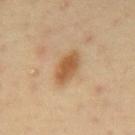follow-up = no biopsy performed (imaged during a skin exam)
lesion diameter = about 4.5 mm
site = the mid back
image-analysis metrics = a within-lesion color-variation index near 3/10; an automated nevus-likeness rating near 90 out of 100 and a lesion-detection confidence of about 100/100
image source = ~15 mm tile from a whole-body skin photo
patient = male, aged 38–42